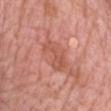Clinical impression:
Imaged during a routine full-body skin examination; the lesion was not biopsied and no histopathology is available.
Image and clinical context:
This is a white-light tile. A 15 mm close-up extracted from a 3D total-body photography capture. On the chest. The subject is a female aged 68–72. The lesion's longest dimension is about 4.5 mm. Automated tile analysis of the lesion measured a lesion color around L≈56 a*≈28 b*≈31 in CIELAB, a lesion–skin lightness drop of about 8, and a lesion-to-skin contrast of about 5.5 (normalized; higher = more distinct). And it measured a classifier nevus-likeness of about 0/100 and lesion-presence confidence of about 100/100.